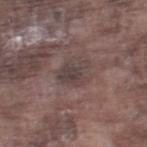No biopsy was performed on this lesion — it was imaged during a full skin examination and was not determined to be concerning. The lesion is on the right thigh. The lesion-visualizer software estimated a footprint of about 6.5 mm², an eccentricity of roughly 0.65, and a shape-asymmetry score of about 0.4 (0 = symmetric). The analysis additionally found border irregularity of about 4 on a 0–10 scale, internal color variation of about 3.5 on a 0–10 scale, and radial color variation of about 1. It also reported a classifier nevus-likeness of about 0/100. This is a white-light tile. A close-up tile cropped from a whole-body skin photograph, about 15 mm across. A male patient, about 75 years old.